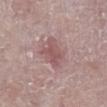The lesion was tiled from a total-body skin photograph and was not biopsied.
A close-up tile cropped from a whole-body skin photograph, about 15 mm across.
Measured at roughly 4 mm in maximum diameter.
A female patient aged 68 to 72.
Located on the right lower leg.
Captured under white-light illumination.